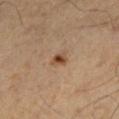Assessment:
Part of a total-body skin-imaging series; this lesion was reviewed on a skin check and was not flagged for biopsy.
Image and clinical context:
A male patient in their mid- to late 50s. The lesion-visualizer software estimated a mean CIELAB color near L≈45 a*≈19 b*≈31, a lesion–skin lightness drop of about 12, and a normalized lesion–skin contrast near 9. Longest diameter approximately 2 mm. A 15 mm close-up extracted from a 3D total-body photography capture. Located on the left lower leg.